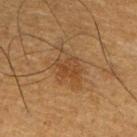The lesion was photographed on a routine skin check and not biopsied; there is no pathology result.